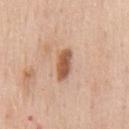Part of a total-body skin-imaging series; this lesion was reviewed on a skin check and was not flagged for biopsy. An algorithmic analysis of the crop reported a lesion area of about 6 mm² and a symmetry-axis asymmetry near 0.15. The analysis additionally found a border-irregularity rating of about 2/10, a color-variation rating of about 2.5/10, and radial color variation of about 1. And it measured a nevus-likeness score of about 100/100 and lesion-presence confidence of about 100/100. A region of skin cropped from a whole-body photographic capture, roughly 15 mm wide. A male patient, approximately 60 years of age. On the chest. Measured at roughly 4 mm in maximum diameter. Imaged with white-light lighting.